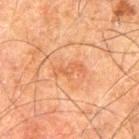Imaged during a routine full-body skin examination; the lesion was not biopsied and no histopathology is available. Approximately 3.5 mm at its widest. A 15 mm close-up extracted from a 3D total-body photography capture. This is a cross-polarized tile. The lesion-visualizer software estimated an area of roughly 4.5 mm² and two-axis asymmetry of about 0.4. The analysis additionally found a border-irregularity rating of about 5.5/10 and radial color variation of about 0. And it measured an automated nevus-likeness rating near 10 out of 100 and a lesion-detection confidence of about 100/100. The lesion is on the left thigh. A male patient aged 78 to 82.